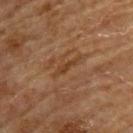  biopsy_status: not biopsied; imaged during a skin examination
  patient:
    sex: male
    age_approx: 65
  image:
    source: total-body photography crop
    field_of_view_mm: 15
  site: upper back
  lighting: cross-polarized